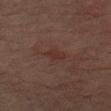{"biopsy_status": "not biopsied; imaged during a skin examination", "image": {"source": "total-body photography crop", "field_of_view_mm": 15}, "site": "left upper arm", "patient": {"sex": "male", "age_approx": 75}}